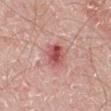TBP lesion metrics — a lesion area of about 6.5 mm², an eccentricity of roughly 0.6, and a shape-asymmetry score of about 0.2 (0 = symmetric); a lesion color around L≈54 a*≈31 b*≈24 in CIELAB, roughly 13 lightness units darker than nearby skin, and a lesion-to-skin contrast of about 8.5 (normalized; higher = more distinct); a border-irregularity index near 1.5/10 and a within-lesion color-variation index near 6.5/10 | subject — male, aged 68 to 72 | site — the right lower leg | image — total-body-photography crop, ~15 mm field of view | lighting — white-light illumination.